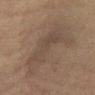Q: Was this lesion biopsied?
A: catalogued during a skin exam; not biopsied
Q: Where on the body is the lesion?
A: the abdomen
Q: How was the tile lit?
A: cross-polarized illumination
Q: How was this image acquired?
A: total-body-photography crop, ~15 mm field of view
Q: Patient demographics?
A: female, about 40 years old
Q: How large is the lesion?
A: ≈7.5 mm